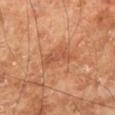The lesion was photographed on a routine skin check and not biopsied; there is no pathology result. Located on the leg. A male subject about 70 years old. A 15 mm close-up extracted from a 3D total-body photography capture.